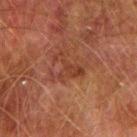Part of a total-body skin-imaging series; this lesion was reviewed on a skin check and was not flagged for biopsy. Automated image analysis of the tile measured a lesion–skin lightness drop of about 6 and a normalized lesion–skin contrast near 6. It also reported a border-irregularity rating of about 8.5/10, internal color variation of about 1.5 on a 0–10 scale, and peripheral color asymmetry of about 0.5. It also reported an automated nevus-likeness rating near 0 out of 100 and a detector confidence of about 100 out of 100 that the crop contains a lesion. Located on the arm. A male subject, aged 73 to 77. A roughly 15 mm field-of-view crop from a total-body skin photograph.